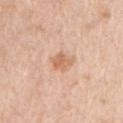Q: Is there a histopathology result?
A: total-body-photography surveillance lesion; no biopsy
Q: What are the patient's age and sex?
A: male, in their mid-50s
Q: How was this image acquired?
A: total-body-photography crop, ~15 mm field of view
Q: How was the tile lit?
A: white-light illumination
Q: Where on the body is the lesion?
A: the chest
Q: What did automated image analysis measure?
A: a footprint of about 4 mm² and a symmetry-axis asymmetry near 0.3; a border-irregularity index near 3/10, a color-variation rating of about 1.5/10, and a peripheral color-asymmetry measure near 0.5